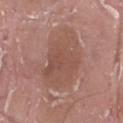Q: Was this lesion biopsied?
A: imaged on a skin check; not biopsied
Q: What is the imaging modality?
A: ~15 mm tile from a whole-body skin photo
Q: Automated lesion metrics?
A: an area of roughly 27 mm² and an eccentricity of roughly 0.85; a lesion color around L≈51 a*≈21 b*≈25 in CIELAB, roughly 7 lightness units darker than nearby skin, and a normalized lesion–skin contrast near 5.5; an automated nevus-likeness rating near 0 out of 100 and lesion-presence confidence of about 100/100
Q: What lighting was used for the tile?
A: white-light
Q: How large is the lesion?
A: about 8 mm
Q: Who is the patient?
A: male, in their 40s
Q: Where on the body is the lesion?
A: the right thigh